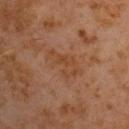Assessment:
The lesion was photographed on a routine skin check and not biopsied; there is no pathology result.
Background:
Measured at roughly 5 mm in maximum diameter. This image is a 15 mm lesion crop taken from a total-body photograph. An algorithmic analysis of the crop reported a lesion area of about 9 mm², an outline eccentricity of about 0.85 (0 = round, 1 = elongated), and two-axis asymmetry of about 0.35. The software also gave a lesion color around L≈41 a*≈20 b*≈31 in CIELAB, roughly 5 lightness units darker than nearby skin, and a normalized border contrast of about 5. The analysis additionally found a border-irregularity rating of about 5.5/10 and a within-lesion color-variation index near 2.5/10. It also reported a nevus-likeness score of about 0/100 and a detector confidence of about 100 out of 100 that the crop contains a lesion. From the chest. The tile uses cross-polarized illumination. A male subject aged approximately 60.